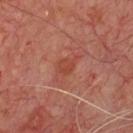follow-up: catalogued during a skin exam; not biopsied
location: the front of the torso
diameter: ~3 mm (longest diameter)
imaging modality: ~15 mm crop, total-body skin-cancer survey
TBP lesion metrics: a mean CIELAB color near L≈44 a*≈29 b*≈30, roughly 6 lightness units darker than nearby skin, and a normalized lesion–skin contrast near 6; a color-variation rating of about 1.5/10 and a peripheral color-asymmetry measure near 0.5; a nevus-likeness score of about 0/100 and a detector confidence of about 100 out of 100 that the crop contains a lesion
patient: male, roughly 65 years of age